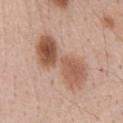notes: no biopsy performed (imaged during a skin exam)
patient: male, approximately 60 years of age
automated lesion analysis: a lesion area of about 24 mm², an outline eccentricity of about 0.9 (0 = round, 1 = elongated), and a symmetry-axis asymmetry near 0.35; a border-irregularity rating of about 4.5/10, a within-lesion color-variation index near 8.5/10, and a peripheral color-asymmetry measure near 3; a classifier nevus-likeness of about 25/100
image source: ~15 mm crop, total-body skin-cancer survey
anatomic site: the abdomen
illumination: white-light
lesion size: ~8 mm (longest diameter)